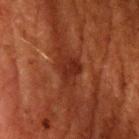The lesion was tiled from a total-body skin photograph and was not biopsied. The lesion's longest dimension is about 3.5 mm. A region of skin cropped from a whole-body photographic capture, roughly 15 mm wide. A male patient about 60 years old. The tile uses cross-polarized illumination. The lesion-visualizer software estimated a border-irregularity index near 3/10. Located on the left upper arm.